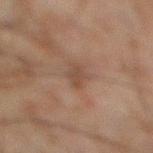Notes:
• notes · catalogued during a skin exam; not biopsied
• location · the right lower leg
• acquisition · ~15 mm tile from a whole-body skin photo
• patient · male, in their mid-40s